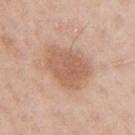Case summary:
– biopsy status · imaged on a skin check; not biopsied
– diameter · about 5.5 mm
– patient · female, approximately 40 years of age
– site · the right upper arm
– acquisition · ~15 mm tile from a whole-body skin photo
– lighting · white-light